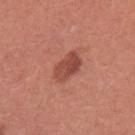Clinical summary:
An algorithmic analysis of the crop reported a border-irregularity rating of about 2/10, a within-lesion color-variation index near 4/10, and radial color variation of about 1.5. And it measured a classifier nevus-likeness of about 75/100. A close-up tile cropped from a whole-body skin photograph, about 15 mm across. The tile uses white-light illumination. From the left upper arm. The patient is a female roughly 25 years of age. Longest diameter approximately 3.5 mm.